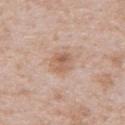Recorded during total-body skin imaging; not selected for excision or biopsy. The patient is a male roughly 65 years of age. The lesion is on the abdomen. The recorded lesion diameter is about 3 mm. A roughly 15 mm field-of-view crop from a total-body skin photograph.